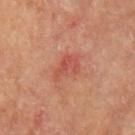Notes:
- notes — catalogued during a skin exam; not biopsied
- lesion size — ≈3.5 mm
- automated lesion analysis — about 7 CIELAB-L* units darker than the surrounding skin; a nevus-likeness score of about 0/100 and a lesion-detection confidence of about 100/100
- image — 15 mm crop, total-body photography
- subject — male, aged around 70
- body site — the left upper arm